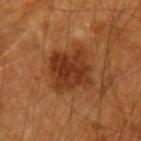Notes:
– biopsy status · total-body-photography surveillance lesion; no biopsy
– anatomic site · the arm
– patient · male, in their mid-50s
– image source · ~15 mm tile from a whole-body skin photo